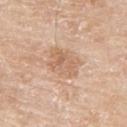Captured during whole-body skin photography for melanoma surveillance; the lesion was not biopsied. A 15 mm close-up tile from a total-body photography series done for melanoma screening. From the right upper arm. A male subject roughly 80 years of age.